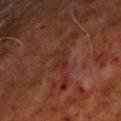Recorded during total-body skin imaging; not selected for excision or biopsy. The lesion is located on the right upper arm. Automated image analysis of the tile measured an eccentricity of roughly 0.8. And it measured a lesion-to-skin contrast of about 5 (normalized; higher = more distinct). The software also gave border irregularity of about 5 on a 0–10 scale and a within-lesion color-variation index near 1/10. Captured under cross-polarized illumination. A male patient in their 70s. Approximately 2.5 mm at its widest. Cropped from a total-body skin-imaging series; the visible field is about 15 mm.